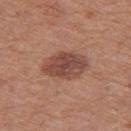| feature | finding |
|---|---|
| follow-up | catalogued during a skin exam; not biopsied |
| illumination | white-light illumination |
| size | ~5 mm (longest diameter) |
| image source | 15 mm crop, total-body photography |
| automated lesion analysis | a classifier nevus-likeness of about 50/100 and a detector confidence of about 100 out of 100 that the crop contains a lesion |
| patient | female, aged around 40 |
| site | the left thigh |